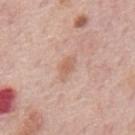Image and clinical context:
A male patient aged 73 to 77. Approximately 2.5 mm at its widest. This is a white-light tile. The lesion is located on the mid back. A region of skin cropped from a whole-body photographic capture, roughly 15 mm wide. Automated tile analysis of the lesion measured a border-irregularity rating of about 3/10, internal color variation of about 1 on a 0–10 scale, and peripheral color asymmetry of about 0.5.